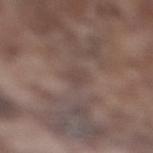Captured during whole-body skin photography for melanoma surveillance; the lesion was not biopsied. A 15 mm close-up tile from a total-body photography series done for melanoma screening. A male subject, about 75 years old. Located on the right lower leg.